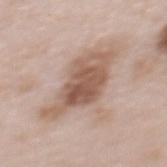Findings:
* follow-up — no biopsy performed (imaged during a skin exam)
* image — 15 mm crop, total-body photography
* patient — female, aged 63 to 67
* tile lighting — white-light
* lesion size — ~8 mm (longest diameter)
* automated metrics — an area of roughly 22 mm² and an eccentricity of roughly 0.85; a lesion color around L≈57 a*≈18 b*≈27 in CIELAB, roughly 12 lightness units darker than nearby skin, and a lesion-to-skin contrast of about 8.5 (normalized; higher = more distinct); a classifier nevus-likeness of about 55/100 and a lesion-detection confidence of about 100/100
* body site — the upper back